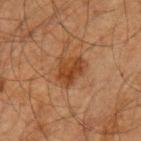| feature | finding |
|---|---|
| workup | imaged on a skin check; not biopsied |
| acquisition | ~15 mm tile from a whole-body skin photo |
| subject | male, in their mid- to late 60s |
| diameter | ≈4 mm |
| tile lighting | cross-polarized |
| anatomic site | the left upper arm |
| TBP lesion metrics | an area of roughly 9.5 mm² and an outline eccentricity of about 0.6 (0 = round, 1 = elongated); peripheral color asymmetry of about 1.5; a nevus-likeness score of about 55/100 and lesion-presence confidence of about 100/100 |